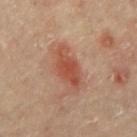{"biopsy_status": "not biopsied; imaged during a skin examination", "lesion_size": {"long_diameter_mm_approx": 5.0}, "lighting": "cross-polarized", "image": {"source": "total-body photography crop", "field_of_view_mm": 15}, "site": "mid back", "patient": {"sex": "male", "age_approx": 65}}